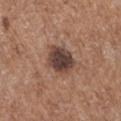| key | value |
|---|---|
| notes | total-body-photography surveillance lesion; no biopsy |
| illumination | white-light |
| patient | male, aged 68–72 |
| body site | the left upper arm |
| acquisition | 15 mm crop, total-body photography |
| diameter | ~4 mm (longest diameter) |